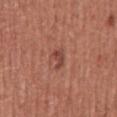Q: Was this lesion biopsied?
A: catalogued during a skin exam; not biopsied
Q: Patient demographics?
A: male, about 45 years old
Q: What kind of image is this?
A: total-body-photography crop, ~15 mm field of view
Q: What is the lesion's diameter?
A: ~2.5 mm (longest diameter)
Q: Lesion location?
A: the abdomen